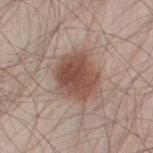workup: catalogued during a skin exam; not biopsied
TBP lesion metrics: a lesion-to-skin contrast of about 9.5 (normalized; higher = more distinct); internal color variation of about 4.5 on a 0–10 scale and a peripheral color-asymmetry measure near 1.5; lesion-presence confidence of about 100/100
image source: ~15 mm tile from a whole-body skin photo
patient: male, aged 33–37
location: the left thigh
diameter: about 5 mm
illumination: white-light illumination